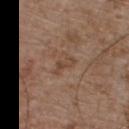imaging modality: total-body-photography crop, ~15 mm field of view | subject: male, in their mid- to late 50s | automated lesion analysis: a footprint of about 3 mm²; a border-irregularity index near 5/10, a color-variation rating of about 0/10, and a peripheral color-asymmetry measure near 0 | lighting: white-light illumination | anatomic site: the chest.